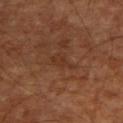| field | value |
|---|---|
| workup | no biopsy performed (imaged during a skin exam) |
| subject | roughly 65 years of age |
| lesion size | ~3 mm (longest diameter) |
| imaging modality | ~15 mm crop, total-body skin-cancer survey |
| site | the right upper arm |
| lighting | cross-polarized illumination |